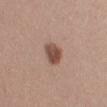Assessment: Captured during whole-body skin photography for melanoma surveillance; the lesion was not biopsied. Acquisition and patient details: This is a white-light tile. A lesion tile, about 15 mm wide, cut from a 3D total-body photograph. Automated tile analysis of the lesion measured two-axis asymmetry of about 0.2. The analysis additionally found about 13 CIELAB-L* units darker than the surrounding skin. And it measured border irregularity of about 2 on a 0–10 scale, a within-lesion color-variation index near 3.5/10, and radial color variation of about 1. The subject is a female aged 38–42. From the right lower leg.